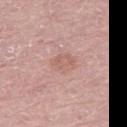Impression:
The lesion was tiled from a total-body skin photograph and was not biopsied.
Context:
A female subject approximately 60 years of age. A 15 mm close-up tile from a total-body photography series done for melanoma screening. On the left thigh.